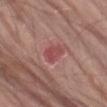Image and clinical context: The subject is a male approximately 80 years of age. A 15 mm close-up tile from a total-body photography series done for melanoma screening. The tile uses white-light illumination. The total-body-photography lesion software estimated a border-irregularity index near 2.5/10, internal color variation of about 3 on a 0–10 scale, and peripheral color asymmetry of about 1. The analysis additionally found an automated nevus-likeness rating near 0 out of 100 and lesion-presence confidence of about 100/100. On the left thigh.